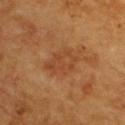biopsy status=imaged on a skin check; not biopsied | patient=male, roughly 65 years of age | body site=the upper back | lighting=cross-polarized | image=~15 mm crop, total-body skin-cancer survey | size=≈4.5 mm.